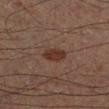site: the left lower leg | patient: male, about 50 years old | acquisition: 15 mm crop, total-body photography.